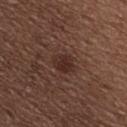Captured during whole-body skin photography for melanoma surveillance; the lesion was not biopsied.
A female subject aged around 65.
The lesion is on the upper back.
A 15 mm close-up extracted from a 3D total-body photography capture.